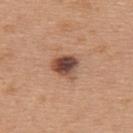Recorded during total-body skin imaging; not selected for excision or biopsy. A 15 mm close-up tile from a total-body photography series done for melanoma screening. The lesion is located on the upper back. A female subject, about 40 years old.